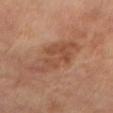Findings:
* follow-up · no biopsy performed (imaged during a skin exam)
* location · the arm
* automated lesion analysis · an average lesion color of about L≈48 a*≈22 b*≈31 (CIELAB) and a lesion–skin lightness drop of about 8; a border-irregularity index near 7.5/10, a color-variation rating of about 3/10, and radial color variation of about 1
* tile lighting · cross-polarized
* subject · female, about 65 years old
* size · ~6.5 mm (longest diameter)
* imaging modality · ~15 mm tile from a whole-body skin photo